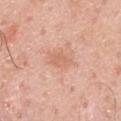Assessment: This lesion was catalogued during total-body skin photography and was not selected for biopsy. Acquisition and patient details: Automated image analysis of the tile measured a footprint of about 4.5 mm², an eccentricity of roughly 0.8, and a symmetry-axis asymmetry near 0.2. The software also gave a normalized lesion–skin contrast near 5. The analysis additionally found a detector confidence of about 100 out of 100 that the crop contains a lesion. The recorded lesion diameter is about 3 mm. A 15 mm close-up tile from a total-body photography series done for melanoma screening. A male patient, approximately 60 years of age. On the mid back. Captured under white-light illumination.